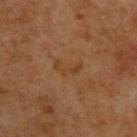notes = total-body-photography surveillance lesion; no biopsy
automated metrics = a lesion area of about 4 mm², a shape eccentricity near 0.85, and two-axis asymmetry of about 0.55
diameter = ~3 mm (longest diameter)
location = the upper back
subject = male, aged 48 to 52
tile lighting = cross-polarized illumination
image source = total-body-photography crop, ~15 mm field of view